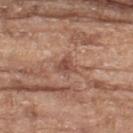Part of a total-body skin-imaging series; this lesion was reviewed on a skin check and was not flagged for biopsy. The subject is a female aged 73 to 77. This image is a 15 mm lesion crop taken from a total-body photograph. Longest diameter approximately 2.5 mm. On the left thigh.